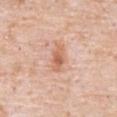biopsy status: imaged on a skin check; not biopsied | size: about 3.5 mm | site: the abdomen | illumination: white-light | patient: male, aged 78–82 | imaging modality: 15 mm crop, total-body photography.